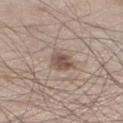No biopsy was performed on this lesion — it was imaged during a full skin examination and was not determined to be concerning. Automated image analysis of the tile measured an automated nevus-likeness rating near 70 out of 100. The patient is a male approximately 60 years of age. The tile uses white-light illumination. The lesion is located on the right lower leg. Cropped from a total-body skin-imaging series; the visible field is about 15 mm. The lesion's longest dimension is about 3 mm.